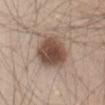Q: Is there a histopathology result?
A: no biopsy performed (imaged during a skin exam)
Q: Lesion location?
A: the abdomen
Q: Who is the patient?
A: male, in their mid-40s
Q: What is the imaging modality?
A: total-body-photography crop, ~15 mm field of view